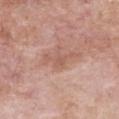Impression: Recorded during total-body skin imaging; not selected for excision or biopsy. Clinical summary: A female subject aged 73 to 77. Located on the chest. The tile uses white-light illumination. Measured at roughly 4.5 mm in maximum diameter. A 15 mm close-up extracted from a 3D total-body photography capture.